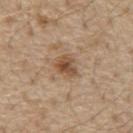Q: Is there a histopathology result?
A: no biopsy performed (imaged during a skin exam)
Q: What did automated image analysis measure?
A: a footprint of about 5.5 mm², an outline eccentricity of about 0.55 (0 = round, 1 = elongated), and two-axis asymmetry of about 0.3; a lesion color around L≈51 a*≈18 b*≈32 in CIELAB, about 11 CIELAB-L* units darker than the surrounding skin, and a normalized lesion–skin contrast near 8; border irregularity of about 2.5 on a 0–10 scale, internal color variation of about 4.5 on a 0–10 scale, and a peripheral color-asymmetry measure near 1.5; a nevus-likeness score of about 75/100 and lesion-presence confidence of about 100/100
Q: Illumination type?
A: white-light illumination
Q: Lesion size?
A: ~3 mm (longest diameter)
Q: What are the patient's age and sex?
A: male, aged around 70
Q: What is the anatomic site?
A: the chest
Q: What is the imaging modality?
A: ~15 mm tile from a whole-body skin photo